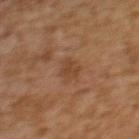Notes:
– workup · catalogued during a skin exam; not biopsied
– location · the upper back
– patient · female, aged 58 to 62
– image source · ~15 mm crop, total-body skin-cancer survey